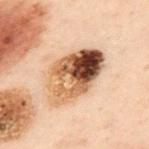biopsy_status: not biopsied; imaged during a skin examination
site: upper back
image:
  source: total-body photography crop
  field_of_view_mm: 15
patient:
  sex: male
  age_approx: 50
lesion_size:
  long_diameter_mm_approx: 7.0